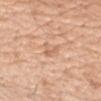Findings:
- workup · imaged on a skin check; not biopsied
- lighting · white-light illumination
- size · ≈2.5 mm
- image source · 15 mm crop, total-body photography
- image-analysis metrics · an area of roughly 3.5 mm², an eccentricity of roughly 0.7, and a symmetry-axis asymmetry near 0.45; a lesion color around L≈65 a*≈21 b*≈35 in CIELAB
- anatomic site · the right upper arm
- subject · female, in their mid-60s